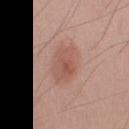biopsy status = imaged on a skin check; not biopsied | site = the arm | automated metrics = a within-lesion color-variation index near 4.5/10 | illumination = white-light illumination | patient = male, about 50 years old | image = total-body-photography crop, ~15 mm field of view.